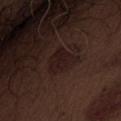Recorded during total-body skin imaging; not selected for excision or biopsy. A male patient, approximately 70 years of age. The lesion is located on the front of the torso. This image is a 15 mm lesion crop taken from a total-body photograph.